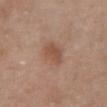No biopsy was performed on this lesion — it was imaged during a full skin examination and was not determined to be concerning. A female patient aged approximately 65. A roughly 15 mm field-of-view crop from a total-body skin photograph. Measured at roughly 3 mm in maximum diameter. The lesion is located on the chest.